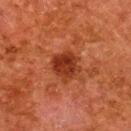* imaging modality — ~15 mm tile from a whole-body skin photo
* anatomic site — the upper back
* subject — female, aged approximately 50
* diameter — ~3.5 mm (longest diameter)